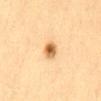Approximately 2.5 mm at its widest. A lesion tile, about 15 mm wide, cut from a 3D total-body photograph. The tile uses cross-polarized illumination. From the mid back. A female subject, aged 28 to 32.